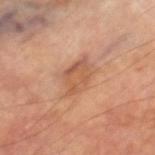Imaged during a routine full-body skin examination; the lesion was not biopsied and no histopathology is available. About 4.5 mm across. From the right thigh. A 15 mm close-up extracted from a 3D total-body photography capture. A male patient aged 68 to 72.